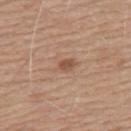Clinical impression:
No biopsy was performed on this lesion — it was imaged during a full skin examination and was not determined to be concerning.
Acquisition and patient details:
The subject is a male aged around 65. Approximately 3 mm at its widest. The lesion is located on the upper back. A 15 mm close-up extracted from a 3D total-body photography capture.